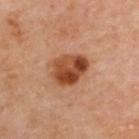Clinical summary: The lesion is located on the back. A 15 mm close-up tile from a total-body photography series done for melanoma screening. Longest diameter approximately 4.5 mm. Automated tile analysis of the lesion measured a mean CIELAB color near L≈47 a*≈26 b*≈36 and a normalized border contrast of about 10.5. And it measured an automated nevus-likeness rating near 95 out of 100 and a detector confidence of about 100 out of 100 that the crop contains a lesion. A female patient approximately 60 years of age.